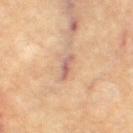{
  "site": "left leg",
  "lighting": "cross-polarized",
  "image": {
    "source": "total-body photography crop",
    "field_of_view_mm": 15
  },
  "patient": {
    "sex": "female",
    "age_approx": 65
  },
  "lesion_size": {
    "long_diameter_mm_approx": 3.0
  },
  "automated_metrics": {
    "cielab_L": 59,
    "cielab_a": 21,
    "cielab_b": 26,
    "vs_skin_darker_L": 10.0,
    "vs_skin_contrast_norm": 8.0,
    "nevus_likeness_0_100": 0,
    "lesion_detection_confidence_0_100": 80
  }
}